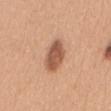The lesion was photographed on a routine skin check and not biopsied; there is no pathology result. A region of skin cropped from a whole-body photographic capture, roughly 15 mm wide. A female patient, roughly 30 years of age. The lesion is on the mid back.